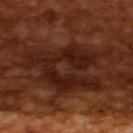Notes:
* biopsy status · total-body-photography surveillance lesion; no biopsy
* patient · male, aged approximately 65
* image source · 15 mm crop, total-body photography